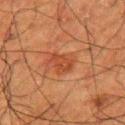Clinical impression: Part of a total-body skin-imaging series; this lesion was reviewed on a skin check and was not flagged for biopsy. Context: Approximately 3.5 mm at its widest. An algorithmic analysis of the crop reported a shape eccentricity near 0.9 and a shape-asymmetry score of about 0.3 (0 = symmetric). The lesion is on the arm. The subject is a male aged around 60. The tile uses cross-polarized illumination. This image is a 15 mm lesion crop taken from a total-body photograph.